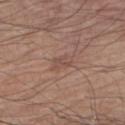subject: male, aged approximately 80
lesion size: ≈3 mm
image-analysis metrics: a footprint of about 4 mm² and an eccentricity of roughly 0.85; a border-irregularity rating of about 3/10, a color-variation rating of about 1.5/10, and radial color variation of about 0.5; an automated nevus-likeness rating near 0 out of 100 and lesion-presence confidence of about 100/100
image: ~15 mm crop, total-body skin-cancer survey
body site: the left arm
tile lighting: white-light